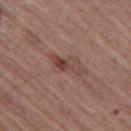Q: Was a biopsy performed?
A: imaged on a skin check; not biopsied
Q: Lesion size?
A: ~4.5 mm (longest diameter)
Q: What is the imaging modality?
A: ~15 mm tile from a whole-body skin photo
Q: Patient demographics?
A: male, approximately 65 years of age
Q: Automated lesion metrics?
A: an average lesion color of about L≈43 a*≈20 b*≈24 (CIELAB) and a lesion–skin lightness drop of about 9
Q: Lesion location?
A: the leg
Q: How was the tile lit?
A: white-light